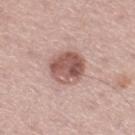This lesion was catalogued during total-body skin photography and was not selected for biopsy. A male patient aged around 65. Located on the right thigh. Automated image analysis of the tile measured a lesion area of about 13 mm², an outline eccentricity of about 0.5 (0 = round, 1 = elongated), and a symmetry-axis asymmetry near 0.1. The analysis additionally found a mean CIELAB color near L≈55 a*≈21 b*≈24. The analysis additionally found a border-irregularity index near 1/10, internal color variation of about 6.5 on a 0–10 scale, and a peripheral color-asymmetry measure near 2.5. Imaged with white-light lighting. A lesion tile, about 15 mm wide, cut from a 3D total-body photograph.